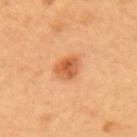This lesion was catalogued during total-body skin photography and was not selected for biopsy. This is a cross-polarized tile. The lesion is located on the upper back. A female subject aged approximately 60. Approximately 3 mm at its widest. This image is a 15 mm lesion crop taken from a total-body photograph.